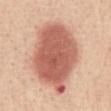Captured during whole-body skin photography for melanoma surveillance; the lesion was not biopsied. Approximately 9.5 mm at its widest. A female subject aged 48–52. Captured under white-light illumination. A close-up tile cropped from a whole-body skin photograph, about 15 mm across. The total-body-photography lesion software estimated a classifier nevus-likeness of about 100/100 and lesion-presence confidence of about 100/100. The lesion is on the front of the torso.